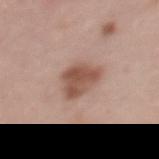Notes:
• follow-up · total-body-photography surveillance lesion; no biopsy
• size · ~4 mm (longest diameter)
• location · the upper back
• acquisition · total-body-photography crop, ~15 mm field of view
• subject · female, aged 38 to 42
• illumination · white-light illumination